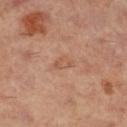Context:
Measured at roughly 2.5 mm in maximum diameter. From the right lower leg. A female subject, aged 58 to 62. Imaged with cross-polarized lighting. A roughly 15 mm field-of-view crop from a total-body skin photograph.